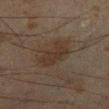<lesion>
  <biopsy_status>not biopsied; imaged during a skin examination</biopsy_status>
  <lesion_size>
    <long_diameter_mm_approx>4.0</long_diameter_mm_approx>
  </lesion_size>
  <lighting>cross-polarized</lighting>
  <patient>
    <sex>male</sex>
    <age_approx>45</age_approx>
  </patient>
  <image>
    <source>total-body photography crop</source>
    <field_of_view_mm>15</field_of_view_mm>
  </image>
  <site>right lower leg</site>
</lesion>